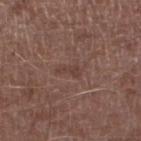Q: Is there a histopathology result?
A: total-body-photography surveillance lesion; no biopsy
Q: What is the anatomic site?
A: the left lower leg
Q: Patient demographics?
A: male, about 45 years old
Q: What is the imaging modality?
A: 15 mm crop, total-body photography